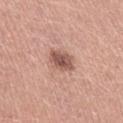size: ≈3 mm | patient: male, aged 28–32 | imaging modality: ~15 mm crop, total-body skin-cancer survey | illumination: white-light illumination | image-analysis metrics: a footprint of about 6.5 mm² and a shape-asymmetry score of about 0.15 (0 = symmetric); an automated nevus-likeness rating near 70 out of 100.